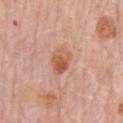{"biopsy_status": "not biopsied; imaged during a skin examination", "site": "front of the torso", "image": {"source": "total-body photography crop", "field_of_view_mm": 15}, "lighting": "white-light", "patient": {"sex": "male", "age_approx": 80}}